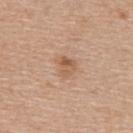Clinical impression:
Captured during whole-body skin photography for melanoma surveillance; the lesion was not biopsied.
Context:
A male subject aged 53 to 57. A close-up tile cropped from a whole-body skin photograph, about 15 mm across. Located on the upper back. Measured at roughly 2.5 mm in maximum diameter. Automated tile analysis of the lesion measured an area of roughly 4.5 mm², an outline eccentricity of about 0.55 (0 = round, 1 = elongated), and two-axis asymmetry of about 0.3. It also reported an average lesion color of about L≈57 a*≈20 b*≈32 (CIELAB) and roughly 9 lightness units darker than nearby skin. And it measured a border-irregularity rating of about 2.5/10 and a color-variation rating of about 5.5/10. Captured under white-light illumination.